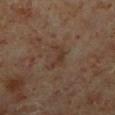The lesion was photographed on a routine skin check and not biopsied; there is no pathology result.
The recorded lesion diameter is about 3.5 mm.
On the right lower leg.
A male patient, aged around 60.
A 15 mm close-up extracted from a 3D total-body photography capture.